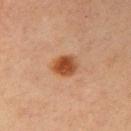Part of a total-body skin-imaging series; this lesion was reviewed on a skin check and was not flagged for biopsy. Approximately 3 mm at its widest. The subject is a female aged 63 to 67. The lesion is located on the right upper arm. This is a cross-polarized tile. Automated image analysis of the tile measured a footprint of about 6.5 mm², an eccentricity of roughly 0.5, and two-axis asymmetry of about 0.15. A 15 mm crop from a total-body photograph taken for skin-cancer surveillance.